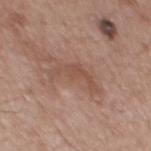{
  "biopsy_status": "not biopsied; imaged during a skin examination",
  "site": "mid back",
  "patient": {
    "sex": "male",
    "age_approx": 55
  },
  "image": {
    "source": "total-body photography crop",
    "field_of_view_mm": 15
  },
  "lighting": "white-light",
  "lesion_size": {
    "long_diameter_mm_approx": 4.5
  }
}